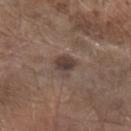Q: Is there a histopathology result?
A: total-body-photography surveillance lesion; no biopsy
Q: What is the lesion's diameter?
A: ~2.5 mm (longest diameter)
Q: What is the imaging modality?
A: ~15 mm crop, total-body skin-cancer survey
Q: Patient demographics?
A: male, roughly 65 years of age
Q: What is the anatomic site?
A: the left forearm
Q: Automated lesion metrics?
A: an eccentricity of roughly 0.35 and a shape-asymmetry score of about 0.35 (0 = symmetric); a lesion–skin lightness drop of about 10 and a normalized border contrast of about 9; border irregularity of about 3.5 on a 0–10 scale, internal color variation of about 2.5 on a 0–10 scale, and a peripheral color-asymmetry measure near 0.5; a classifier nevus-likeness of about 85/100 and a lesion-detection confidence of about 100/100